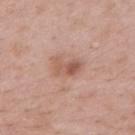This lesion was catalogued during total-body skin photography and was not selected for biopsy.
A 15 mm close-up tile from a total-body photography series done for melanoma screening.
Located on the mid back.
About 3.5 mm across.
Captured under white-light illumination.
A male subject aged approximately 55.